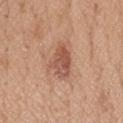notes: no biopsy performed (imaged during a skin exam) | acquisition: ~15 mm crop, total-body skin-cancer survey | tile lighting: white-light illumination | TBP lesion metrics: an area of roughly 8 mm², a shape eccentricity near 0.9, and two-axis asymmetry of about 0.4; border irregularity of about 4 on a 0–10 scale, a color-variation rating of about 4/10, and a peripheral color-asymmetry measure near 1.5; an automated nevus-likeness rating near 25 out of 100 and lesion-presence confidence of about 100/100 | body site: the head or neck | patient: male, roughly 20 years of age.